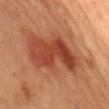| feature | finding |
|---|---|
| biopsy status | total-body-photography surveillance lesion; no biopsy |
| image source | total-body-photography crop, ~15 mm field of view |
| body site | the back |
| patient | female, about 60 years old |